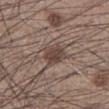workup = imaged on a skin check; not biopsied | lighting = white-light illumination | automated metrics = an average lesion color of about L≈43 a*≈15 b*≈21 (CIELAB), about 10 CIELAB-L* units darker than the surrounding skin, and a normalized lesion–skin contrast near 8 | body site = the left lower leg | image = ~15 mm tile from a whole-body skin photo | subject = male, in their mid-30s.